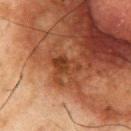{
  "biopsy_status": "not biopsied; imaged during a skin examination",
  "automated_metrics": {
    "cielab_L": 32,
    "cielab_a": 22,
    "cielab_b": 29,
    "vs_skin_darker_L": 8.0,
    "lesion_detection_confidence_0_100": 100
  },
  "image": {
    "source": "total-body photography crop",
    "field_of_view_mm": 15
  },
  "site": "chest",
  "lighting": "cross-polarized",
  "patient": {
    "sex": "male",
    "age_approx": 75
  }
}